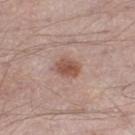Clinical impression:
Captured during whole-body skin photography for melanoma surveillance; the lesion was not biopsied.
Clinical summary:
On the right thigh. A male subject aged 53–57. A 15 mm close-up tile from a total-body photography series done for melanoma screening.